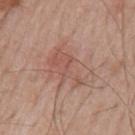Findings:
– follow-up · imaged on a skin check; not biopsied
– image · total-body-photography crop, ~15 mm field of view
– subject · male, in their 70s
– anatomic site · the arm